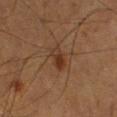Impression:
No biopsy was performed on this lesion — it was imaged during a full skin examination and was not determined to be concerning.
Background:
Longest diameter approximately 3 mm. Imaged with cross-polarized lighting. The patient is a male aged 53 to 57. Cropped from a whole-body photographic skin survey; the tile spans about 15 mm. Located on the right lower leg.